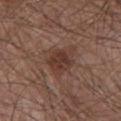Part of a total-body skin-imaging series; this lesion was reviewed on a skin check and was not flagged for biopsy. The lesion-visualizer software estimated a border-irregularity rating of about 3.5/10 and a peripheral color-asymmetry measure near 1.5. A 15 mm crop from a total-body photograph taken for skin-cancer surveillance. Approximately 4.5 mm at its widest. A male subject approximately 65 years of age. Located on the front of the torso.